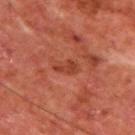<lesion>
<biopsy_status>not biopsied; imaged during a skin examination</biopsy_status>
<site>upper back</site>
<patient>
  <sex>male</sex>
  <age_approx>65</age_approx>
</patient>
<image>
  <source>total-body photography crop</source>
  <field_of_view_mm>15</field_of_view_mm>
</image>
<automated_metrics>
  <cielab_L>37</cielab_L>
  <cielab_a>29</cielab_a>
  <cielab_b>30</cielab_b>
  <vs_skin_contrast_norm>6.0</vs_skin_contrast_norm>
</automated_metrics>
</lesion>